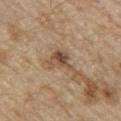Assessment:
Captured during whole-body skin photography for melanoma surveillance; the lesion was not biopsied.
Image and clinical context:
A 15 mm close-up extracted from a 3D total-body photography capture. The lesion is located on the chest. A male subject, about 70 years old. This is a white-light tile. The lesion's longest dimension is about 3.5 mm. Automated image analysis of the tile measured a lesion color around L≈49 a*≈16 b*≈31 in CIELAB, roughly 11 lightness units darker than nearby skin, and a lesion-to-skin contrast of about 8 (normalized; higher = more distinct). The software also gave internal color variation of about 7 on a 0–10 scale.